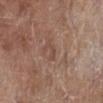Background:
A close-up tile cropped from a whole-body skin photograph, about 15 mm across. A female patient aged around 80. Located on the right lower leg. This is a white-light tile. The recorded lesion diameter is about 2.5 mm.